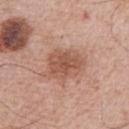patient: male, aged approximately 65 | site: the right upper arm | image-analysis metrics: a mean CIELAB color near L≈55 a*≈22 b*≈29, roughly 10 lightness units darker than nearby skin, and a normalized border contrast of about 7; an automated nevus-likeness rating near 65 out of 100 and a detector confidence of about 100 out of 100 that the crop contains a lesion | image source: 15 mm crop, total-body photography.